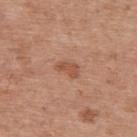Context:
Captured under white-light illumination. The subject is a female in their 40s. The total-body-photography lesion software estimated a mean CIELAB color near L≈53 a*≈23 b*≈32, a lesion–skin lightness drop of about 8, and a normalized border contrast of about 6. A lesion tile, about 15 mm wide, cut from a 3D total-body photograph. Measured at roughly 3 mm in maximum diameter. Located on the upper back.